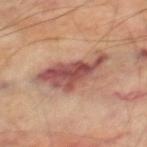Imaged during a routine full-body skin examination; the lesion was not biopsied and no histopathology is available. On the right thigh. A male patient approximately 70 years of age. Longest diameter approximately 8 mm. This is a cross-polarized tile. The total-body-photography lesion software estimated a mean CIELAB color near L≈51 a*≈23 b*≈25 and about 13 CIELAB-L* units darker than the surrounding skin. A 15 mm close-up extracted from a 3D total-body photography capture.